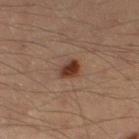This lesion was catalogued during total-body skin photography and was not selected for biopsy. Automated image analysis of the tile measured a border-irregularity index near 2/10, a color-variation rating of about 4.5/10, and a peripheral color-asymmetry measure near 1.5. The lesion is located on the right thigh. A lesion tile, about 15 mm wide, cut from a 3D total-body photograph. Measured at roughly 2.5 mm in maximum diameter. A male subject, aged 38–42.